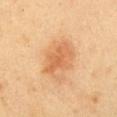Assessment: Part of a total-body skin-imaging series; this lesion was reviewed on a skin check and was not flagged for biopsy. Background: A female subject aged around 30. This image is a 15 mm lesion crop taken from a total-body photograph. Approximately 4.5 mm at its widest. The lesion is located on the chest. The tile uses cross-polarized illumination. Automated tile analysis of the lesion measured a mean CIELAB color near L≈58 a*≈23 b*≈38 and about 9 CIELAB-L* units darker than the surrounding skin. It also reported a border-irregularity index near 3/10 and a within-lesion color-variation index near 3/10. And it measured a nevus-likeness score of about 25/100 and lesion-presence confidence of about 100/100.